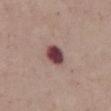biopsy_status: not biopsied; imaged during a skin examination
site: abdomen
image:
  source: total-body photography crop
  field_of_view_mm: 15
patient:
  sex: male
  age_approx: 55